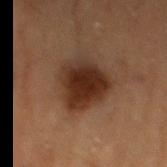notes = total-body-photography surveillance lesion; no biopsy
site = the left thigh
tile lighting = cross-polarized
size = ≈5 mm
subject = male, in their mid-80s or older
automated lesion analysis = a footprint of about 17 mm², a shape eccentricity near 0.55, and two-axis asymmetry of about 0.25; an average lesion color of about L≈25 a*≈17 b*≈24 (CIELAB), a lesion–skin lightness drop of about 12, and a normalized lesion–skin contrast near 12; a border-irregularity index near 2.5/10, a color-variation rating of about 4/10, and a peripheral color-asymmetry measure near 1
image source = ~15 mm tile from a whole-body skin photo